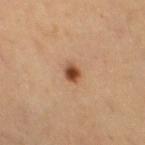Findings:
• biopsy status · total-body-photography surveillance lesion; no biopsy
• automated metrics · border irregularity of about 1 on a 0–10 scale, a within-lesion color-variation index near 3.5/10, and radial color variation of about 1; a nevus-likeness score of about 100/100 and lesion-presence confidence of about 100/100
• lesion diameter · ~2 mm (longest diameter)
• image source · ~15 mm crop, total-body skin-cancer survey
• site · the back
• patient · male, roughly 65 years of age